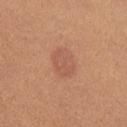Recorded during total-body skin imaging; not selected for excision or biopsy.